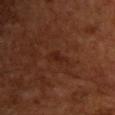Q: Patient demographics?
A: male, aged 53 to 57
Q: How was this image acquired?
A: 15 mm crop, total-body photography
Q: Where on the body is the lesion?
A: the chest